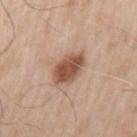Notes:
- follow-up · total-body-photography surveillance lesion; no biopsy